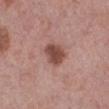Acquisition and patient details:
The subject is a female in their 40s. This is a white-light tile. The lesion is on the right lower leg. A region of skin cropped from a whole-body photographic capture, roughly 15 mm wide. The lesion's longest dimension is about 3 mm.